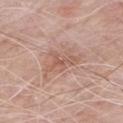Findings:
• follow-up: no biopsy performed (imaged during a skin exam)
• tile lighting: white-light illumination
• body site: the chest
• image source: ~15 mm tile from a whole-body skin photo
• subject: male, in their 80s
• lesion diameter: ~4 mm (longest diameter)
• image-analysis metrics: a lesion area of about 5.5 mm², an eccentricity of roughly 0.8, and a shape-asymmetry score of about 0.65 (0 = symmetric); roughly 8 lightness units darker than nearby skin and a normalized border contrast of about 5.5